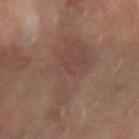<record>
  <biopsy_status>not biopsied; imaged during a skin examination</biopsy_status>
  <lesion_size>
    <long_diameter_mm_approx>9.5</long_diameter_mm_approx>
  </lesion_size>
  <site>left forearm</site>
  <image>
    <source>total-body photography crop</source>
    <field_of_view_mm>15</field_of_view_mm>
  </image>
  <lighting>cross-polarized</lighting>
  <patient>
    <sex>female</sex>
    <age_approx>60</age_approx>
  </patient>
</record>